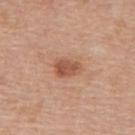  biopsy_status: not biopsied; imaged during a skin examination
  automated_metrics:
    border_irregularity_0_10: 2.5
    color_variation_0_10: 3.0
    peripheral_color_asymmetry: 1.0
    nevus_likeness_0_100: 80
    lesion_detection_confidence_0_100: 100
  lighting: white-light
  patient:
    sex: female
    age_approx: 40
  site: back
  image:
    source: total-body photography crop
    field_of_view_mm: 15